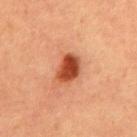acquisition: total-body-photography crop, ~15 mm field of view
image-analysis metrics: border irregularity of about 2 on a 0–10 scale, a within-lesion color-variation index near 5/10, and radial color variation of about 1.5; an automated nevus-likeness rating near 100 out of 100 and lesion-presence confidence of about 100/100
diameter: about 3.5 mm
patient: male, aged approximately 65
anatomic site: the abdomen
illumination: cross-polarized